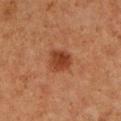Impression:
Imaged during a routine full-body skin examination; the lesion was not biopsied and no histopathology is available.